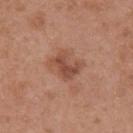Q: What is the anatomic site?
A: the upper back
Q: What is the imaging modality?
A: ~15 mm crop, total-body skin-cancer survey
Q: What are the patient's age and sex?
A: female, in their 40s